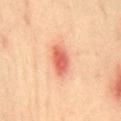A close-up tile cropped from a whole-body skin photograph, about 15 mm across. About 4 mm across. Imaged with cross-polarized lighting. A female patient aged 38–42. Automated tile analysis of the lesion measured two-axis asymmetry of about 0.2. And it measured a lesion color around L≈65 a*≈33 b*≈35 in CIELAB, roughly 14 lightness units darker than nearby skin, and a lesion-to-skin contrast of about 8.5 (normalized; higher = more distinct). And it measured a classifier nevus-likeness of about 90/100 and a lesion-detection confidence of about 100/100. Located on the mid back.